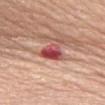follow-up: catalogued during a skin exam; not biopsied | anatomic site: the front of the torso | image source: ~15 mm tile from a whole-body skin photo | lighting: white-light illumination | subject: female, aged around 65 | lesion size: about 3.5 mm.